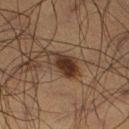<case>
<biopsy_status>not biopsied; imaged during a skin examination</biopsy_status>
<site>right thigh</site>
<patient>
  <sex>male</sex>
  <age_approx>50</age_approx>
</patient>
<lesion_size>
  <long_diameter_mm_approx>4.5</long_diameter_mm_approx>
</lesion_size>
<automated_metrics>
  <area_mm2_approx>7.5</area_mm2_approx>
  <eccentricity>0.85</eccentricity>
  <shape_asymmetry>0.25</shape_asymmetry>
  <cielab_L>25</cielab_L>
  <cielab_a>13</cielab_a>
  <cielab_b>21</cielab_b>
  <vs_skin_darker_L>10.0</vs_skin_darker_L>
  <vs_skin_contrast_norm>11.0</vs_skin_contrast_norm>
  <border_irregularity_0_10>2.5</border_irregularity_0_10>
  <color_variation_0_10>5.5</color_variation_0_10>
  <peripheral_color_asymmetry>2.0</peripheral_color_asymmetry>
</automated_metrics>
<image>
  <source>total-body photography crop</source>
  <field_of_view_mm>15</field_of_view_mm>
</image>
</case>